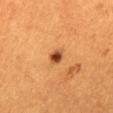imaging modality = 15 mm crop, total-body photography
subject = female, aged around 55
diameter = about 2.5 mm
body site = the back
illumination = cross-polarized